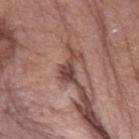Impression:
The lesion was photographed on a routine skin check and not biopsied; there is no pathology result.
Context:
The total-body-photography lesion software estimated a footprint of about 4 mm² and a shape-asymmetry score of about 0.6 (0 = symmetric). A male subject, aged 73–77. The lesion is on the left forearm. A 15 mm close-up tile from a total-body photography series done for melanoma screening.